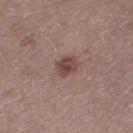The lesion was photographed on a routine skin check and not biopsied; there is no pathology result.
On the leg.
The lesion's longest dimension is about 2.5 mm.
Automated image analysis of the tile measured a mean CIELAB color near L≈45 a*≈18 b*≈22, about 11 CIELAB-L* units darker than the surrounding skin, and a normalized lesion–skin contrast near 8.5.
Captured under white-light illumination.
A region of skin cropped from a whole-body photographic capture, roughly 15 mm wide.
The patient is a female in their 50s.